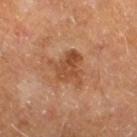The lesion was photographed on a routine skin check and not biopsied; there is no pathology result.
Located on the right leg.
This is a cross-polarized tile.
The lesion's longest dimension is about 5 mm.
Cropped from a total-body skin-imaging series; the visible field is about 15 mm.
A male patient aged 58 to 62.